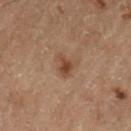Part of a total-body skin-imaging series; this lesion was reviewed on a skin check and was not flagged for biopsy.
The lesion is on the left thigh.
The subject is a male about 70 years old.
A 15 mm close-up tile from a total-body photography series done for melanoma screening.